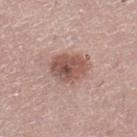Impression: Captured during whole-body skin photography for melanoma surveillance; the lesion was not biopsied. Acquisition and patient details: Longest diameter approximately 4.5 mm. The lesion is located on the leg. A lesion tile, about 15 mm wide, cut from a 3D total-body photograph. The tile uses white-light illumination. A female subject in their 40s. Automated tile analysis of the lesion measured a nevus-likeness score of about 80/100 and a lesion-detection confidence of about 100/100.